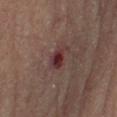<case>
<biopsy_status>not biopsied; imaged during a skin examination</biopsy_status>
<lesion_size>
  <long_diameter_mm_approx>3.0</long_diameter_mm_approx>
</lesion_size>
<lighting>cross-polarized</lighting>
<image>
  <source>total-body photography crop</source>
  <field_of_view_mm>15</field_of_view_mm>
</image>
<automated_metrics>
  <area_mm2_approx>5.0</area_mm2_approx>
  <eccentricity>0.8</eccentricity>
  <shape_asymmetry>0.3</shape_asymmetry>
  <border_irregularity_0_10>3.0</border_irregularity_0_10>
  <color_variation_0_10>5.0</color_variation_0_10>
  <peripheral_color_asymmetry>1.5</peripheral_color_asymmetry>
</automated_metrics>
<site>arm</site>
<patient>
  <sex>male</sex>
  <age_approx>65</age_approx>
</patient>
</case>